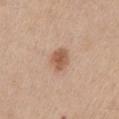<case>
  <image>
    <source>total-body photography crop</source>
    <field_of_view_mm>15</field_of_view_mm>
  </image>
  <lesion_size>
    <long_diameter_mm_approx>3.0</long_diameter_mm_approx>
  </lesion_size>
  <site>arm</site>
  <automated_metrics>
    <area_mm2_approx>5.0</area_mm2_approx>
    <shape_asymmetry>0.2</shape_asymmetry>
    <cielab_L>56</cielab_L>
    <cielab_a>21</cielab_a>
    <cielab_b>31</cielab_b>
    <vs_skin_contrast_norm>8.0</vs_skin_contrast_norm>
    <nevus_likeness_0_100>90</nevus_likeness_0_100>
  </automated_metrics>
  <patient>
    <sex>male</sex>
    <age_approx>60</age_approx>
  </patient>
  <lighting>white-light</lighting>
</case>